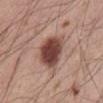Impression: Recorded during total-body skin imaging; not selected for excision or biopsy. Background: The patient is a male approximately 55 years of age. The lesion is on the front of the torso. A 15 mm close-up extracted from a 3D total-body photography capture.